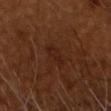Impression: Part of a total-body skin-imaging series; this lesion was reviewed on a skin check and was not flagged for biopsy. Context: On the head or neck. A male subject, aged around 55. Longest diameter approximately 3.5 mm. A 15 mm close-up extracted from a 3D total-body photography capture. The tile uses cross-polarized illumination.